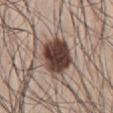Captured during whole-body skin photography for melanoma surveillance; the lesion was not biopsied. Located on the abdomen. The recorded lesion diameter is about 5.5 mm. A male patient roughly 25 years of age. A region of skin cropped from a whole-body photographic capture, roughly 15 mm wide. The total-body-photography lesion software estimated a lesion color around L≈41 a*≈16 b*≈22 in CIELAB, a lesion–skin lightness drop of about 20, and a normalized border contrast of about 14.5. It also reported internal color variation of about 6 on a 0–10 scale. And it measured a nevus-likeness score of about 100/100 and lesion-presence confidence of about 100/100.